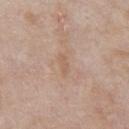Located on the chest. Automated tile analysis of the lesion measured a mean CIELAB color near L≈59 a*≈17 b*≈29, roughly 6 lightness units darker than nearby skin, and a lesion-to-skin contrast of about 4.5 (normalized; higher = more distinct). A male subject, aged approximately 65. A lesion tile, about 15 mm wide, cut from a 3D total-body photograph.